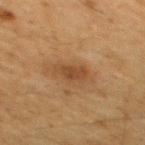Impression:
No biopsy was performed on this lesion — it was imaged during a full skin examination and was not determined to be concerning.
Background:
A male subject, aged 58–62. On the mid back. About 3 mm across. Imaged with cross-polarized lighting. The lesion-visualizer software estimated a footprint of about 4.5 mm², an eccentricity of roughly 0.75, and two-axis asymmetry of about 0.25. And it measured a lesion–skin lightness drop of about 7 and a lesion-to-skin contrast of about 6.5 (normalized; higher = more distinct). The software also gave border irregularity of about 2.5 on a 0–10 scale, a color-variation rating of about 2/10, and a peripheral color-asymmetry measure near 0.5. The software also gave an automated nevus-likeness rating near 15 out of 100 and a detector confidence of about 100 out of 100 that the crop contains a lesion. Cropped from a total-body skin-imaging series; the visible field is about 15 mm.